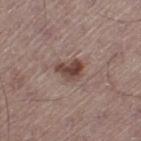Clinical impression: Recorded during total-body skin imaging; not selected for excision or biopsy. Clinical summary: The lesion-visualizer software estimated a mean CIELAB color near L≈44 a*≈18 b*≈22 and a normalized lesion–skin contrast near 9.5. The analysis additionally found a nevus-likeness score of about 90/100. Imaged with white-light lighting. A close-up tile cropped from a whole-body skin photograph, about 15 mm across. A male subject, roughly 55 years of age. The lesion is on the left thigh.